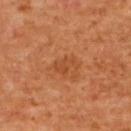Impression:
Recorded during total-body skin imaging; not selected for excision or biopsy.
Background:
This image is a 15 mm lesion crop taken from a total-body photograph. This is a cross-polarized tile. About 3 mm across. The subject is a female in their mid-60s. The lesion is on the upper back.